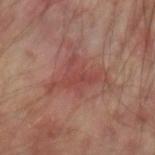{
  "biopsy_status": "not biopsied; imaged during a skin examination",
  "lighting": "cross-polarized",
  "lesion_size": {
    "long_diameter_mm_approx": 6.0
  },
  "image": {
    "source": "total-body photography crop",
    "field_of_view_mm": 15
  },
  "patient": {
    "age_approx": 55
  },
  "site": "left forearm",
  "automated_metrics": {
    "cielab_L": 45,
    "cielab_a": 26,
    "cielab_b": 24,
    "vs_skin_darker_L": 7.0,
    "nevus_likeness_0_100": 5
  }
}